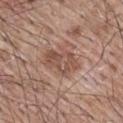Clinical impression:
Recorded during total-body skin imaging; not selected for excision or biopsy.
Background:
The subject is a male about 65 years old. Automated image analysis of the tile measured an average lesion color of about L≈50 a*≈20 b*≈26 (CIELAB) and roughly 9 lightness units darker than nearby skin. And it measured internal color variation of about 4 on a 0–10 scale and a peripheral color-asymmetry measure near 1.5. Longest diameter approximately 4.5 mm. From the mid back. Imaged with white-light lighting. A 15 mm close-up tile from a total-body photography series done for melanoma screening.